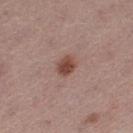Imaged during a routine full-body skin examination; the lesion was not biopsied and no histopathology is available. Captured under white-light illumination. A roughly 15 mm field-of-view crop from a total-body skin photograph. On the left thigh. The patient is a female about 55 years old.